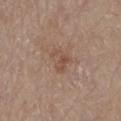workup — catalogued during a skin exam; not biopsied
patient — male, in their 80s
site — the right arm
image — ~15 mm crop, total-body skin-cancer survey